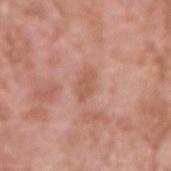Q: Was this lesion biopsied?
A: total-body-photography surveillance lesion; no biopsy
Q: How was this image acquired?
A: total-body-photography crop, ~15 mm field of view
Q: What is the anatomic site?
A: the right upper arm
Q: What are the patient's age and sex?
A: male, roughly 60 years of age